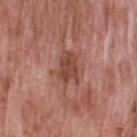Q: Is there a histopathology result?
A: total-body-photography surveillance lesion; no biopsy
Q: What lighting was used for the tile?
A: white-light illumination
Q: Patient demographics?
A: male, in their 60s
Q: What is the anatomic site?
A: the upper back
Q: Automated lesion metrics?
A: a lesion color around L≈47 a*≈24 b*≈28 in CIELAB, about 10 CIELAB-L* units darker than the surrounding skin, and a lesion-to-skin contrast of about 7.5 (normalized; higher = more distinct); a border-irregularity index near 4/10, internal color variation of about 3 on a 0–10 scale, and peripheral color asymmetry of about 1; a nevus-likeness score of about 0/100 and lesion-presence confidence of about 100/100
Q: How was this image acquired?
A: total-body-photography crop, ~15 mm field of view
Q: How large is the lesion?
A: ≈4 mm